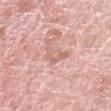No biopsy was performed on this lesion — it was imaged during a full skin examination and was not determined to be concerning.
Automated tile analysis of the lesion measured an area of roughly 4 mm², an outline eccentricity of about 0.9 (0 = round, 1 = elongated), and a shape-asymmetry score of about 0.5 (0 = symmetric). And it measured about 8 CIELAB-L* units darker than the surrounding skin and a normalized border contrast of about 5. It also reported a nevus-likeness score of about 0/100 and a detector confidence of about 85 out of 100 that the crop contains a lesion.
The lesion is located on the left forearm.
A female subject, aged 58–62.
A region of skin cropped from a whole-body photographic capture, roughly 15 mm wide.
Measured at roughly 3.5 mm in maximum diameter.